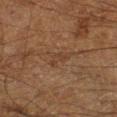This lesion was catalogued during total-body skin photography and was not selected for biopsy.
This image is a 15 mm lesion crop taken from a total-body photograph.
A male subject, roughly 60 years of age.
About 2.5 mm across.
Imaged with cross-polarized lighting.
From the right lower leg.